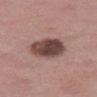Notes:
– notes · no biopsy performed (imaged during a skin exam)
– subject · female, in their mid- to late 40s
– image · total-body-photography crop, ~15 mm field of view
– anatomic site · the right thigh
– tile lighting · white-light illumination